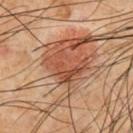{"biopsy_status": "not biopsied; imaged during a skin examination", "image": {"source": "total-body photography crop", "field_of_view_mm": 15}, "site": "chest", "patient": {"sex": "male", "age_approx": 65}, "automated_metrics": {"area_mm2_approx": 9.5, "eccentricity": 0.7, "shape_asymmetry": 0.6, "nevus_likeness_0_100": 100, "lesion_detection_confidence_0_100": 95}, "lesion_size": {"long_diameter_mm_approx": 4.5}, "lighting": "cross-polarized"}